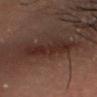follow-up: total-body-photography surveillance lesion; no biopsy
image: total-body-photography crop, ~15 mm field of view
subject: male, in their 30s
site: the head or neck